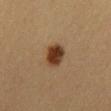follow-up — total-body-photography surveillance lesion; no biopsy.